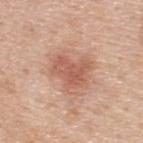illumination — white-light
image — ~15 mm tile from a whole-body skin photo
size — ≈4.5 mm
anatomic site — the upper back
subject — male, approximately 50 years of age